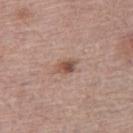follow-up = no biopsy performed (imaged during a skin exam) | subject = female, approximately 65 years of age | image = 15 mm crop, total-body photography | lesion diameter = ~2.5 mm (longest diameter) | TBP lesion metrics = a shape eccentricity near 0.7 and two-axis asymmetry of about 0.25; a border-irregularity rating of about 2.5/10, a within-lesion color-variation index near 5/10, and radial color variation of about 2 | body site = the right thigh.